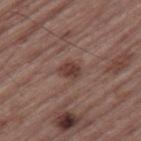Part of a total-body skin-imaging series; this lesion was reviewed on a skin check and was not flagged for biopsy. A 15 mm close-up tile from a total-body photography series done for melanoma screening. The subject is a male approximately 65 years of age. The lesion is located on the leg. The tile uses white-light illumination. The lesion-visualizer software estimated an average lesion color of about L≈39 a*≈20 b*≈23 (CIELAB), about 10 CIELAB-L* units darker than the surrounding skin, and a normalized border contrast of about 8.5. The analysis additionally found border irregularity of about 2 on a 0–10 scale, internal color variation of about 2.5 on a 0–10 scale, and a peripheral color-asymmetry measure near 1. The lesion's longest dimension is about 2.5 mm.